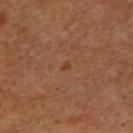Notes:
– notes — imaged on a skin check; not biopsied
– acquisition — total-body-photography crop, ~15 mm field of view
– subject — male, about 60 years old
– body site — the arm